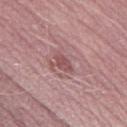Imaged during a routine full-body skin examination; the lesion was not biopsied and no histopathology is available. A male patient aged 48–52. A roughly 15 mm field-of-view crop from a total-body skin photograph. Imaged with white-light lighting. The lesion is located on the leg. Automated image analysis of the tile measured a lesion color around L≈50 a*≈25 b*≈19 in CIELAB, a lesion–skin lightness drop of about 9, and a lesion-to-skin contrast of about 6.5 (normalized; higher = more distinct). It also reported a border-irregularity index near 2.5/10 and a peripheral color-asymmetry measure near 0.5. About 2.5 mm across.